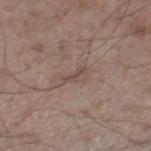Notes:
• biopsy status — catalogued during a skin exam; not biopsied
• automated metrics — an outline eccentricity of about 0.9 (0 = round, 1 = elongated) and a symmetry-axis asymmetry near 0.4; an automated nevus-likeness rating near 0 out of 100 and a detector confidence of about 85 out of 100 that the crop contains a lesion
• subject — male, aged 53–57
• lesion size — ~2.5 mm (longest diameter)
• body site — the right thigh
• imaging modality — 15 mm crop, total-body photography